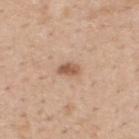notes=imaged on a skin check; not biopsied | lesion size=about 2.5 mm | subject=male, approximately 60 years of age | site=the back | TBP lesion metrics=a border-irregularity index near 2/10, a within-lesion color-variation index near 2/10, and radial color variation of about 0.5 | acquisition=~15 mm tile from a whole-body skin photo | lighting=white-light illumination.